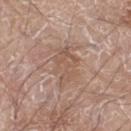biopsy status = total-body-photography surveillance lesion; no biopsy | subject = male, aged around 80 | body site = the left leg | diameter = about 5 mm | image = ~15 mm tile from a whole-body skin photo | lighting = white-light.